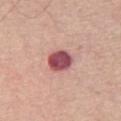Part of a total-body skin-imaging series; this lesion was reviewed on a skin check and was not flagged for biopsy. The tile uses white-light illumination. The lesion is located on the leg. An algorithmic analysis of the crop reported a footprint of about 7 mm². And it measured a lesion–skin lightness drop of about 19 and a lesion-to-skin contrast of about 13 (normalized; higher = more distinct). The software also gave a border-irregularity rating of about 1.5/10, internal color variation of about 3.5 on a 0–10 scale, and a peripheral color-asymmetry measure near 1. It also reported a classifier nevus-likeness of about 10/100 and lesion-presence confidence of about 100/100. A roughly 15 mm field-of-view crop from a total-body skin photograph. Measured at roughly 3 mm in maximum diameter. A male patient, approximately 70 years of age.